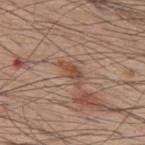workup = total-body-photography surveillance lesion; no biopsy
size = ≈3.5 mm
body site = the upper back
imaging modality = total-body-photography crop, ~15 mm field of view
illumination = white-light
patient = male, about 60 years old